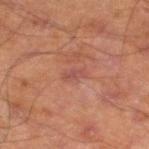No biopsy was performed on this lesion — it was imaged during a full skin examination and was not determined to be concerning.
A male patient, approximately 70 years of age.
The lesion is on the left lower leg.
Approximately 2.5 mm at its widest.
A 15 mm close-up tile from a total-body photography series done for melanoma screening.
The total-body-photography lesion software estimated an average lesion color of about L≈40 a*≈22 b*≈23 (CIELAB). The software also gave a peripheral color-asymmetry measure near 0.